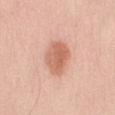Clinical impression: No biopsy was performed on this lesion — it was imaged during a full skin examination and was not determined to be concerning. Acquisition and patient details: The lesion is located on the abdomen. Cropped from a whole-body photographic skin survey; the tile spans about 15 mm. Measured at roughly 4 mm in maximum diameter. A male patient, aged around 70. Imaged with white-light lighting. Automated image analysis of the tile measured a mean CIELAB color near L≈63 a*≈25 b*≈32, roughly 11 lightness units darker than nearby skin, and a lesion-to-skin contrast of about 7.5 (normalized; higher = more distinct). And it measured a border-irregularity rating of about 2/10, a within-lesion color-variation index near 3.5/10, and peripheral color asymmetry of about 1. The analysis additionally found lesion-presence confidence of about 100/100.